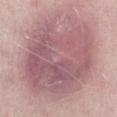Notes:
- workup: total-body-photography surveillance lesion; no biopsy
- subject: female, in their mid- to late 40s
- tile lighting: white-light illumination
- site: the left lower leg
- imaging modality: 15 mm crop, total-body photography
- image-analysis metrics: a lesion area of about 75 mm², an eccentricity of roughly 0.5, and two-axis asymmetry of about 0.15; border irregularity of about 3 on a 0–10 scale, internal color variation of about 6 on a 0–10 scale, and a peripheral color-asymmetry measure near 2
- lesion diameter: about 10.5 mm